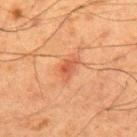notes = imaged on a skin check; not biopsied
patient = male, aged 58 to 62
diameter = about 3 mm
image source = total-body-photography crop, ~15 mm field of view
location = the upper back
illumination = cross-polarized